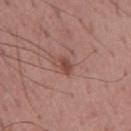Impression:
Imaged during a routine full-body skin examination; the lesion was not biopsied and no histopathology is available.
Background:
The total-body-photography lesion software estimated a footprint of about 2.5 mm², an outline eccentricity of about 0.85 (0 = round, 1 = elongated), and two-axis asymmetry of about 0.4. The analysis additionally found a lesion–skin lightness drop of about 10. The analysis additionally found an automated nevus-likeness rating near 65 out of 100 and lesion-presence confidence of about 100/100. Captured under white-light illumination. The lesion is located on the mid back. The subject is a male approximately 55 years of age. Cropped from a total-body skin-imaging series; the visible field is about 15 mm.